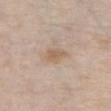Imaged during a routine full-body skin examination; the lesion was not biopsied and no histopathology is available. The lesion is located on the chest. A female patient, approximately 65 years of age. A region of skin cropped from a whole-body photographic capture, roughly 15 mm wide.